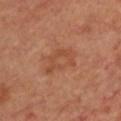Assessment:
Part of a total-body skin-imaging series; this lesion was reviewed on a skin check and was not flagged for biopsy.
Image and clinical context:
Cropped from a total-body skin-imaging series; the visible field is about 15 mm. Located on the chest. The patient is a female in their mid- to late 40s. An algorithmic analysis of the crop reported an area of roughly 9.5 mm², a shape eccentricity near 0.75, and a symmetry-axis asymmetry near 0.3. The software also gave an average lesion color of about L≈47 a*≈24 b*≈32 (CIELAB), about 6 CIELAB-L* units darker than the surrounding skin, and a normalized lesion–skin contrast near 5. The software also gave a border-irregularity index near 3.5/10 and a color-variation rating of about 3/10. The lesion's longest dimension is about 4.5 mm. Imaged with cross-polarized lighting.